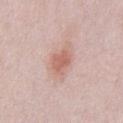A male subject aged 23 to 27. The lesion is on the chest. The total-body-photography lesion software estimated a shape eccentricity near 0.7 and a shape-asymmetry score of about 0.25 (0 = symmetric). The software also gave a mean CIELAB color near L≈62 a*≈23 b*≈27, a lesion–skin lightness drop of about 10, and a lesion-to-skin contrast of about 7 (normalized; higher = more distinct). About 3.5 mm across. Captured under white-light illumination. This image is a 15 mm lesion crop taken from a total-body photograph.